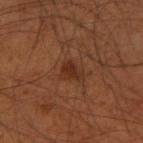workup = imaged on a skin check; not biopsied
tile lighting = cross-polarized illumination
automated metrics = a border-irregularity rating of about 4/10 and peripheral color asymmetry of about 1; a nevus-likeness score of about 70/100 and a detector confidence of about 100 out of 100 that the crop contains a lesion
diameter = ≈3 mm
subject = male, roughly 35 years of age
acquisition = ~15 mm crop, total-body skin-cancer survey
body site = the right upper arm